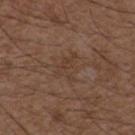Impression: The lesion was photographed on a routine skin check and not biopsied; there is no pathology result. Context: From the chest. A male patient, roughly 50 years of age. A close-up tile cropped from a whole-body skin photograph, about 15 mm across. Automated image analysis of the tile measured a classifier nevus-likeness of about 0/100 and a detector confidence of about 95 out of 100 that the crop contains a lesion. This is a white-light tile. About 3.5 mm across.